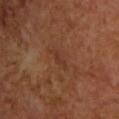{
  "image": {
    "source": "total-body photography crop",
    "field_of_view_mm": 15
  },
  "lighting": "cross-polarized",
  "lesion_size": {
    "long_diameter_mm_approx": 2.5
  },
  "site": "chest",
  "patient": {
    "sex": "male",
    "age_approx": 70
  }
}